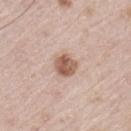Context: Longest diameter approximately 3 mm. On the left thigh. The tile uses white-light illumination. Cropped from a total-body skin-imaging series; the visible field is about 15 mm. The subject is a male aged 78–82.